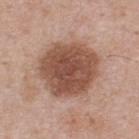Image and clinical context:
A male patient, aged 43–47. The lesion is on the upper back. A roughly 15 mm field-of-view crop from a total-body skin photograph.